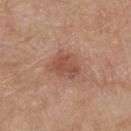Q: What is the imaging modality?
A: ~15 mm crop, total-body skin-cancer survey
Q: Patient demographics?
A: male, aged 78 to 82
Q: What is the anatomic site?
A: the chest